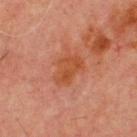biopsy status — catalogued during a skin exam; not biopsied | anatomic site — the chest | size — ~4 mm (longest diameter) | subject — male, approximately 70 years of age | acquisition — ~15 mm crop, total-body skin-cancer survey.